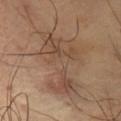Case summary:
* workup · total-body-photography surveillance lesion; no biopsy
* acquisition · total-body-photography crop, ~15 mm field of view
* site · the abdomen
* lighting · cross-polarized illumination
* diameter · ≈8 mm
* patient · female, about 50 years old